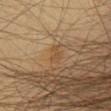{
  "biopsy_status": "not biopsied; imaged during a skin examination",
  "site": "chest",
  "image": {
    "source": "total-body photography crop",
    "field_of_view_mm": 15
  },
  "patient": {
    "sex": "male",
    "age_approx": 55
  }
}